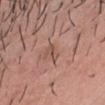This lesion was catalogued during total-body skin photography and was not selected for biopsy.
Imaged with white-light lighting.
A roughly 15 mm field-of-view crop from a total-body skin photograph.
The lesion-visualizer software estimated a footprint of about 2.5 mm², an outline eccentricity of about 0.95 (0 = round, 1 = elongated), and a symmetry-axis asymmetry near 0.45. And it measured an average lesion color of about L≈51 a*≈19 b*≈26 (CIELAB) and roughly 8 lightness units darker than nearby skin.
Approximately 2.5 mm at its widest.
The lesion is located on the chest.
The patient is a male aged 33 to 37.